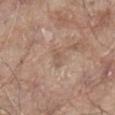follow-up = imaged on a skin check; not biopsied
patient = male, aged approximately 80
image = ~15 mm tile from a whole-body skin photo
anatomic site = the abdomen